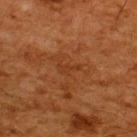- biopsy status · imaged on a skin check; not biopsied
- image-analysis metrics · a border-irregularity rating of about 6/10, a within-lesion color-variation index near 0/10, and a peripheral color-asymmetry measure near 0; a detector confidence of about 100 out of 100 that the crop contains a lesion
- lighting · cross-polarized illumination
- site · the upper back
- image source · 15 mm crop, total-body photography
- subject · male, aged around 65
- lesion size · ~2.5 mm (longest diameter)